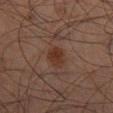Case summary:
• workup — total-body-photography surveillance lesion; no biopsy
• image — 15 mm crop, total-body photography
• diameter — ~2.5 mm (longest diameter)
• lighting — cross-polarized illumination
• patient — male, roughly 70 years of age
• body site — the leg